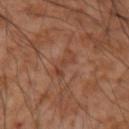The tile uses cross-polarized illumination.
The lesion's longest dimension is about 3.5 mm.
A male patient roughly 55 years of age.
A 15 mm crop from a total-body photograph taken for skin-cancer surveillance.
The lesion is on the right forearm.